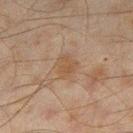Imaged during a routine full-body skin examination; the lesion was not biopsied and no histopathology is available. About 3 mm across. This is a cross-polarized tile. An algorithmic analysis of the crop reported roughly 5 lightness units darker than nearby skin. The analysis additionally found internal color variation of about 1.5 on a 0–10 scale and radial color variation of about 0.5. The software also gave an automated nevus-likeness rating near 0 out of 100 and a detector confidence of about 100 out of 100 that the crop contains a lesion. A close-up tile cropped from a whole-body skin photograph, about 15 mm across. The lesion is located on the right thigh. A male patient approximately 45 years of age.